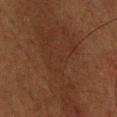Q: Lesion location?
A: the chest
Q: How large is the lesion?
A: ~14 mm (longest diameter)
Q: Patient demographics?
A: male, aged around 60
Q: What kind of image is this?
A: ~15 mm tile from a whole-body skin photo